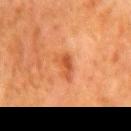Case summary:
- follow-up · imaged on a skin check; not biopsied
- acquisition · ~15 mm crop, total-body skin-cancer survey
- subject · male, about 80 years old
- lesion diameter · ≈2.5 mm
- lighting · cross-polarized
- anatomic site · the mid back